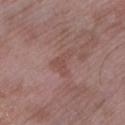biopsy status: imaged on a skin check; not biopsied
tile lighting: white-light
TBP lesion metrics: a footprint of about 4.5 mm², an eccentricity of roughly 0.75, and a shape-asymmetry score of about 0.65 (0 = symmetric); a mean CIELAB color near L≈48 a*≈21 b*≈22, roughly 6 lightness units darker than nearby skin, and a normalized border contrast of about 5; a border-irregularity rating of about 6.5/10 and a peripheral color-asymmetry measure near 0.5; a detector confidence of about 100 out of 100 that the crop contains a lesion
acquisition: ~15 mm tile from a whole-body skin photo
anatomic site: the left lower leg
lesion diameter: ≈3.5 mm
patient: female, aged 68 to 72